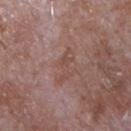biopsy status: no biopsy performed (imaged during a skin exam) | image-analysis metrics: an outline eccentricity of about 0.95 (0 = round, 1 = elongated); a mean CIELAB color near L≈48 a*≈20 b*≈24, a lesion–skin lightness drop of about 6, and a normalized border contrast of about 5 | site: the chest | size: ≈4 mm | subject: male, aged 63 to 67 | image: 15 mm crop, total-body photography | illumination: white-light.